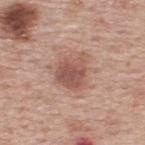Case summary:
* workup: catalogued during a skin exam; not biopsied
* subject: male, in their mid-70s
* TBP lesion metrics: an area of roughly 11 mm² and a shape eccentricity near 0.45; a lesion-to-skin contrast of about 7.5 (normalized; higher = more distinct); a border-irregularity rating of about 3/10, a within-lesion color-variation index near 3.5/10, and a peripheral color-asymmetry measure near 1
* lesion size: ~4 mm (longest diameter)
* imaging modality: 15 mm crop, total-body photography
* location: the upper back
* lighting: white-light illumination